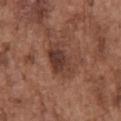Findings:
- location — the front of the torso
- patient — male, about 75 years old
- lighting — white-light
- TBP lesion metrics — a lesion color around L≈38 a*≈20 b*≈25 in CIELAB, a lesion–skin lightness drop of about 9, and a normalized lesion–skin contrast near 8; a lesion-detection confidence of about 100/100
- acquisition — ~15 mm tile from a whole-body skin photo